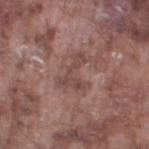Clinical impression:
This lesion was catalogued during total-body skin photography and was not selected for biopsy.
Clinical summary:
Captured under white-light illumination. Cropped from a whole-body photographic skin survey; the tile spans about 15 mm. The patient is a male approximately 75 years of age. On the left thigh. About 4 mm across.